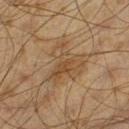Imaged during a routine full-body skin examination; the lesion was not biopsied and no histopathology is available.
Cropped from a whole-body photographic skin survey; the tile spans about 15 mm.
On the left thigh.
A male subject, in their 60s.